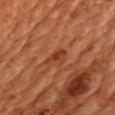Q: Was a biopsy performed?
A: imaged on a skin check; not biopsied
Q: Lesion location?
A: the chest
Q: Patient demographics?
A: male, aged around 60
Q: How was this image acquired?
A: ~15 mm tile from a whole-body skin photo